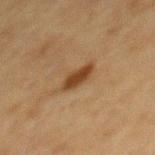| feature | finding |
|---|---|
| image source | ~15 mm crop, total-body skin-cancer survey |
| anatomic site | the mid back |
| patient | male, aged approximately 75 |